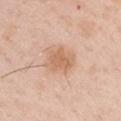Part of a total-body skin-imaging series; this lesion was reviewed on a skin check and was not flagged for biopsy.
The lesion-visualizer software estimated a lesion color around L≈64 a*≈20 b*≈33 in CIELAB and a lesion–skin lightness drop of about 9. The analysis additionally found a border-irregularity index near 2.5/10 and a within-lesion color-variation index near 2.5/10. The analysis additionally found an automated nevus-likeness rating near 35 out of 100.
The lesion's longest dimension is about 3.5 mm.
Imaged with white-light lighting.
From the arm.
A 15 mm close-up tile from a total-body photography series done for melanoma screening.
A male subject aged around 50.